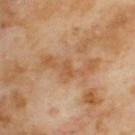Imaged during a routine full-body skin examination; the lesion was not biopsied and no histopathology is available.
A male subject, aged 68 to 72.
Captured under cross-polarized illumination.
The lesion-visualizer software estimated a footprint of about 8.5 mm². The software also gave internal color variation of about 2 on a 0–10 scale and radial color variation of about 0.5. The analysis additionally found lesion-presence confidence of about 100/100.
A close-up tile cropped from a whole-body skin photograph, about 15 mm across.
Located on the back.
Approximately 6.5 mm at its widest.